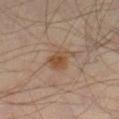biopsy status=imaged on a skin check; not biopsied | body site=the right thigh | imaging modality=~15 mm tile from a whole-body skin photo | subject=male, about 45 years old.